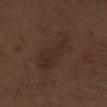Q: Is there a histopathology result?
A: imaged on a skin check; not biopsied
Q: What are the patient's age and sex?
A: male, aged 68–72
Q: What is the anatomic site?
A: the right thigh
Q: Automated lesion metrics?
A: an area of roughly 18 mm²; border irregularity of about 4.5 on a 0–10 scale and a peripheral color-asymmetry measure near 1; an automated nevus-likeness rating near 15 out of 100 and lesion-presence confidence of about 100/100
Q: Lesion size?
A: ≈7.5 mm
Q: What lighting was used for the tile?
A: white-light illumination
Q: How was this image acquired?
A: total-body-photography crop, ~15 mm field of view